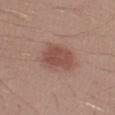| key | value |
|---|---|
| biopsy status | no biopsy performed (imaged during a skin exam) |
| body site | the left forearm |
| subject | male, aged around 30 |
| automated metrics | a mean CIELAB color near L≈49 a*≈22 b*≈24, roughly 9 lightness units darker than nearby skin, and a lesion-to-skin contrast of about 7 (normalized; higher = more distinct); border irregularity of about 2.5 on a 0–10 scale, a color-variation rating of about 3/10, and peripheral color asymmetry of about 1 |
| imaging modality | 15 mm crop, total-body photography |
| size | ≈4 mm |
| lighting | white-light |